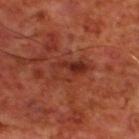Findings:
* follow-up: no biopsy performed (imaged during a skin exam)
* location: the upper back
* size: ≈4.5 mm
* imaging modality: 15 mm crop, total-body photography
* TBP lesion metrics: an average lesion color of about L≈32 a*≈28 b*≈29 (CIELAB) and a lesion–skin lightness drop of about 8
* tile lighting: cross-polarized illumination
* subject: male, aged around 70